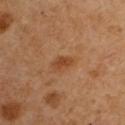notes — no biopsy performed (imaged during a skin exam)